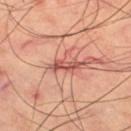Imaged during a routine full-body skin examination; the lesion was not biopsied and no histopathology is available. The lesion is located on the leg. A male subject roughly 65 years of age. A region of skin cropped from a whole-body photographic capture, roughly 15 mm wide. This is a cross-polarized tile. Automated image analysis of the tile measured an average lesion color of about L≈53 a*≈29 b*≈28 (CIELAB), a lesion–skin lightness drop of about 13, and a normalized border contrast of about 8.5. And it measured a color-variation rating of about 0/10 and radial color variation of about 0.